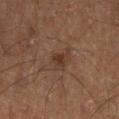  site: left lower leg
  lighting: cross-polarized
  image:
    source: total-body photography crop
    field_of_view_mm: 15
  lesion_size:
    long_diameter_mm_approx: 2.5
  patient:
    sex: male
    age_approx: 50